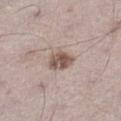Clinical impression:
Captured during whole-body skin photography for melanoma surveillance; the lesion was not biopsied.
Acquisition and patient details:
Captured under white-light illumination. A 15 mm close-up extracted from a 3D total-body photography capture. A male subject, approximately 70 years of age. On the left lower leg.